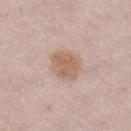Part of a total-body skin-imaging series; this lesion was reviewed on a skin check and was not flagged for biopsy. This image is a 15 mm lesion crop taken from a total-body photograph. The lesion's longest dimension is about 3.5 mm. The lesion is located on the right lower leg. A female patient, in their 20s. Captured under white-light illumination. Automated image analysis of the tile measured an area of roughly 10 mm² and an outline eccentricity of about 0.3 (0 = round, 1 = elongated). It also reported about 9 CIELAB-L* units darker than the surrounding skin and a lesion-to-skin contrast of about 7 (normalized; higher = more distinct).